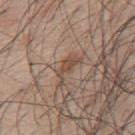Imaged with white-light lighting.
A close-up tile cropped from a whole-body skin photograph, about 15 mm across.
A male subject, roughly 55 years of age.
The lesion-visualizer software estimated a shape eccentricity near 0.9 and two-axis asymmetry of about 0.45. And it measured roughly 9 lightness units darker than nearby skin and a lesion-to-skin contrast of about 6.5 (normalized; higher = more distinct). The analysis additionally found a color-variation rating of about 1.5/10 and radial color variation of about 0.5.
From the upper back.